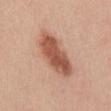The lesion was tiled from a total-body skin photograph and was not biopsied. Automated tile analysis of the lesion measured an area of roughly 17 mm² and a symmetry-axis asymmetry near 0.15. A female patient, roughly 45 years of age. A close-up tile cropped from a whole-body skin photograph, about 15 mm across. The lesion is on the mid back. About 7.5 mm across.